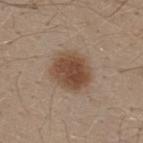workup: catalogued during a skin exam; not biopsied
illumination: white-light illumination
acquisition: total-body-photography crop, ~15 mm field of view
site: the upper back
patient: male, aged 28–32